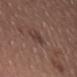Captured during whole-body skin photography for melanoma surveillance; the lesion was not biopsied.
A female patient, aged around 25.
Cropped from a whole-body photographic skin survey; the tile spans about 15 mm.
The lesion is located on the left thigh.
About 3 mm across.